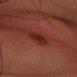A 15 mm close-up extracted from a 3D total-body photography capture.
Automated image analysis of the tile measured a mean CIELAB color near L≈23 a*≈25 b*≈23 and roughly 7 lightness units darker than nearby skin. It also reported a nevus-likeness score of about 45/100 and lesion-presence confidence of about 95/100.
A female patient about 45 years old.
The lesion is on the head or neck.
Longest diameter approximately 3 mm.